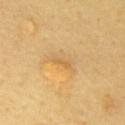Assessment:
The lesion was tiled from a total-body skin photograph and was not biopsied.
Acquisition and patient details:
About 2 mm across. This is a cross-polarized tile. This image is a 15 mm lesion crop taken from a total-body photograph. The lesion is on the chest. A male patient approximately 65 years of age.